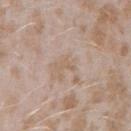Q: Is there a histopathology result?
A: total-body-photography surveillance lesion; no biopsy
Q: Lesion size?
A: ~2.5 mm (longest diameter)
Q: Illumination type?
A: white-light illumination
Q: Patient demographics?
A: female, aged 23 to 27
Q: How was this image acquired?
A: ~15 mm crop, total-body skin-cancer survey
Q: What did automated image analysis measure?
A: a lesion–skin lightness drop of about 6 and a normalized lesion–skin contrast near 5; a color-variation rating of about 0/10 and a peripheral color-asymmetry measure near 0; an automated nevus-likeness rating near 0 out of 100 and a lesion-detection confidence of about 90/100
Q: What is the anatomic site?
A: the right forearm